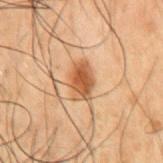Assessment: This lesion was catalogued during total-body skin photography and was not selected for biopsy. Image and clinical context: Located on the mid back. A 15 mm close-up tile from a total-body photography series done for melanoma screening. Longest diameter approximately 4 mm. This is a cross-polarized tile. The subject is a male approximately 50 years of age.